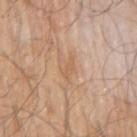No biopsy was performed on this lesion — it was imaged during a full skin examination and was not determined to be concerning. From the right upper arm. A close-up tile cropped from a whole-body skin photograph, about 15 mm across. Imaged with white-light lighting. A male patient, in their 80s. An algorithmic analysis of the crop reported an eccentricity of roughly 0.9. And it measured a lesion color around L≈60 a*≈19 b*≈34 in CIELAB and a lesion-to-skin contrast of about 4.5 (normalized; higher = more distinct). The analysis additionally found a nevus-likeness score of about 0/100 and a detector confidence of about 100 out of 100 that the crop contains a lesion. The lesion's longest dimension is about 2.5 mm.